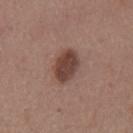Part of a total-body skin-imaging series; this lesion was reviewed on a skin check and was not flagged for biopsy. About 4.5 mm across. On the back. Captured under white-light illumination. A 15 mm close-up extracted from a 3D total-body photography capture. The subject is a male approximately 55 years of age. Automated tile analysis of the lesion measured an area of roughly 8.5 mm², an outline eccentricity of about 0.85 (0 = round, 1 = elongated), and a shape-asymmetry score of about 0.15 (0 = symmetric). The analysis additionally found a lesion–skin lightness drop of about 13 and a normalized border contrast of about 10.